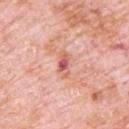follow-up: total-body-photography surveillance lesion; no biopsy
lesion diameter: ~3 mm (longest diameter)
patient: male, aged around 80
automated lesion analysis: a border-irregularity index near 4/10, a color-variation rating of about 5.5/10, and radial color variation of about 1; a nevus-likeness score of about 0/100 and a detector confidence of about 100 out of 100 that the crop contains a lesion
image source: ~15 mm crop, total-body skin-cancer survey
location: the upper back